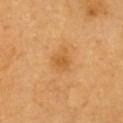Findings:
* notes · imaged on a skin check; not biopsied
* location · the chest
* lighting · cross-polarized
* patient · male, roughly 60 years of age
* imaging modality · 15 mm crop, total-body photography
* diameter · ~2.5 mm (longest diameter)